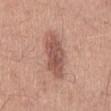The lesion was photographed on a routine skin check and not biopsied; there is no pathology result. A male subject aged approximately 30. Longest diameter approximately 7 mm. A roughly 15 mm field-of-view crop from a total-body skin photograph. From the mid back. Automated image analysis of the tile measured an outline eccentricity of about 0.95 (0 = round, 1 = elongated). It also reported a lesion color around L≈54 a*≈22 b*≈26 in CIELAB, roughly 12 lightness units darker than nearby skin, and a normalized border contrast of about 8. The software also gave a classifier nevus-likeness of about 75/100 and a lesion-detection confidence of about 100/100. Captured under white-light illumination.